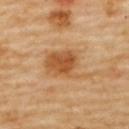biopsy status: catalogued during a skin exam; not biopsied | imaging modality: ~15 mm tile from a whole-body skin photo | lighting: cross-polarized | patient: female, aged 58 to 62 | TBP lesion metrics: a footprint of about 14 mm², a shape eccentricity near 0.7, and a shape-asymmetry score of about 0.35 (0 = symmetric); a border-irregularity index near 4.5/10 and internal color variation of about 4.5 on a 0–10 scale; an automated nevus-likeness rating near 90 out of 100 | site: the upper back | lesion diameter: ≈6 mm.